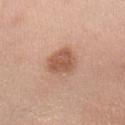Captured during whole-body skin photography for melanoma surveillance; the lesion was not biopsied. From the arm. Cropped from a whole-body photographic skin survey; the tile spans about 15 mm. A female patient aged 48 to 52.